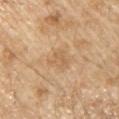biopsy status: total-body-photography surveillance lesion; no biopsy | patient: male, aged around 70 | size: ≈2.5 mm | image source: 15 mm crop, total-body photography | illumination: white-light illumination | TBP lesion metrics: a mean CIELAB color near L≈62 a*≈17 b*≈37, about 6 CIELAB-L* units darker than the surrounding skin, and a lesion-to-skin contrast of about 4.5 (normalized; higher = more distinct); border irregularity of about 4 on a 0–10 scale; an automated nevus-likeness rating near 0 out of 100 and lesion-presence confidence of about 100/100 | location: the left upper arm.